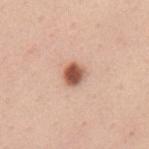• notes · total-body-photography surveillance lesion; no biopsy
• patient · female, aged approximately 40
• image source · ~15 mm tile from a whole-body skin photo
• tile lighting · white-light illumination
• anatomic site · the mid back